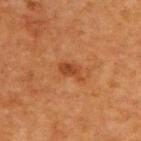follow-up: catalogued during a skin exam; not biopsied | lesion size: about 3 mm | image: ~15 mm crop, total-body skin-cancer survey | patient: male, in their mid-60s | location: the back | illumination: cross-polarized illumination | image-analysis metrics: an outline eccentricity of about 0.85 (0 = round, 1 = elongated); a mean CIELAB color near L≈39 a*≈25 b*≈36 and a normalized border contrast of about 7; a border-irregularity rating of about 4/10, a within-lesion color-variation index near 2/10, and radial color variation of about 0.5; a nevus-likeness score of about 55/100.